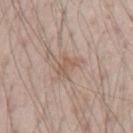Impression: Captured during whole-body skin photography for melanoma surveillance; the lesion was not biopsied. Clinical summary: From the left upper arm. Longest diameter approximately 3 mm. A male subject, approximately 70 years of age. Cropped from a total-body skin-imaging series; the visible field is about 15 mm. Captured under white-light illumination.